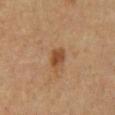Findings:
* biopsy status · no biopsy performed (imaged during a skin exam)
* image · ~15 mm crop, total-body skin-cancer survey
* TBP lesion metrics · a lesion area of about 4.5 mm², an outline eccentricity of about 0.65 (0 = round, 1 = elongated), and a shape-asymmetry score of about 0.2 (0 = symmetric)
* size · ≈2.5 mm
* patient · male, in their 60s
* tile lighting · cross-polarized illumination
* location · the mid back